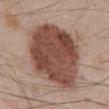Clinical impression:
The lesion was tiled from a total-body skin photograph and was not biopsied.
Acquisition and patient details:
A 15 mm crop from a total-body photograph taken for skin-cancer surveillance. The lesion is on the abdomen. A male patient, in their mid-40s.